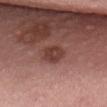notes = imaged on a skin check; not biopsied | anatomic site = the lower back | lesion size = ≈3 mm | illumination = white-light | acquisition = ~15 mm crop, total-body skin-cancer survey | patient = male, aged around 40 | automated metrics = a footprint of about 6 mm², a shape eccentricity near 0.55, and a symmetry-axis asymmetry near 0.2; a mean CIELAB color near L≈39 a*≈23 b*≈24 and a lesion–skin lightness drop of about 9.